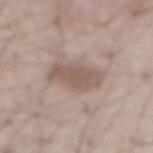Impression: Captured during whole-body skin photography for melanoma surveillance; the lesion was not biopsied. Acquisition and patient details: Captured under white-light illumination. The lesion is on the abdomen. Automated tile analysis of the lesion measured an area of roughly 11 mm², an outline eccentricity of about 0.75 (0 = round, 1 = elongated), and two-axis asymmetry of about 0.25. The lesion's longest dimension is about 4.5 mm. A 15 mm close-up tile from a total-body photography series done for melanoma screening. A male patient roughly 55 years of age.